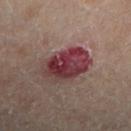Recorded during total-body skin imaging; not selected for excision or biopsy.
A close-up tile cropped from a whole-body skin photograph, about 15 mm across.
Measured at roughly 5 mm in maximum diameter.
Located on the leg.
Captured under cross-polarized illumination.
A female subject, in their mid- to late 50s.